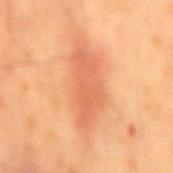Clinical impression: The lesion was photographed on a routine skin check and not biopsied; there is no pathology result. Acquisition and patient details: A close-up tile cropped from a whole-body skin photograph, about 15 mm across. The lesion is located on the mid back. A male patient aged 63–67.